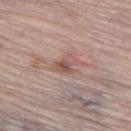  biopsy_status: not biopsied; imaged during a skin examination
  image:
    source: total-body photography crop
    field_of_view_mm: 15
  patient:
    sex: male
    age_approx: 80
  site: leg
  lighting: white-light